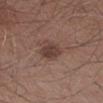Clinical impression: Captured during whole-body skin photography for melanoma surveillance; the lesion was not biopsied. Image and clinical context: From the left forearm. Longest diameter approximately 3 mm. This is a white-light tile. The patient is a male in their mid-50s. This image is a 15 mm lesion crop taken from a total-body photograph.